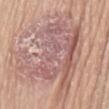Imaged during a routine full-body skin examination; the lesion was not biopsied and no histopathology is available. On the abdomen. This image is a 15 mm lesion crop taken from a total-body photograph. Captured under white-light illumination. The patient is a male aged 63–67.